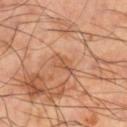Q: Is there a histopathology result?
A: total-body-photography surveillance lesion; no biopsy
Q: Lesion location?
A: the right lower leg
Q: What are the patient's age and sex?
A: male, aged approximately 50
Q: What is the imaging modality?
A: total-body-photography crop, ~15 mm field of view